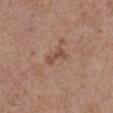<record>
<biopsy_status>not biopsied; imaged during a skin examination</biopsy_status>
<image>
  <source>total-body photography crop</source>
  <field_of_view_mm>15</field_of_view_mm>
</image>
<patient>
  <sex>female</sex>
  <age_approx>65</age_approx>
</patient>
<automated_metrics>
  <nevus_likeness_0_100>0</nevus_likeness_0_100>
  <lesion_detection_confidence_0_100>100</lesion_detection_confidence_0_100>
</automated_metrics>
<site>abdomen</site>
</record>